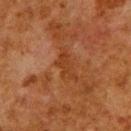Clinical impression:
Imaged during a routine full-body skin examination; the lesion was not biopsied and no histopathology is available.
Background:
The lesion is located on the upper back. The subject is a male about 80 years old. The recorded lesion diameter is about 4 mm. A 15 mm close-up tile from a total-body photography series done for melanoma screening. This is a cross-polarized tile.